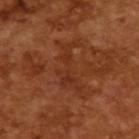The lesion was tiled from a total-body skin photograph and was not biopsied. Captured under cross-polarized illumination. Automated image analysis of the tile measured a lesion color around L≈32 a*≈25 b*≈32 in CIELAB. The software also gave an automated nevus-likeness rating near 0 out of 100 and a lesion-detection confidence of about 90/100. A roughly 15 mm field-of-view crop from a total-body skin photograph. The patient is a male aged 63 to 67.